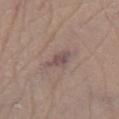This lesion was catalogued during total-body skin photography and was not selected for biopsy.
The lesion is on the left lower leg.
A male patient, in their mid-60s.
A lesion tile, about 15 mm wide, cut from a 3D total-body photograph.
Captured under white-light illumination.
The total-body-photography lesion software estimated a footprint of about 4.5 mm², an eccentricity of roughly 0.9, and a symmetry-axis asymmetry near 0.25. And it measured a mean CIELAB color near L≈49 a*≈17 b*≈17, about 9 CIELAB-L* units darker than the surrounding skin, and a normalized lesion–skin contrast near 7.5.
Approximately 3.5 mm at its widest.